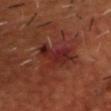Clinical impression:
No biopsy was performed on this lesion — it was imaged during a full skin examination and was not determined to be concerning.
Acquisition and patient details:
A male patient, aged 48–52. A lesion tile, about 15 mm wide, cut from a 3D total-body photograph. The lesion is located on the head or neck.